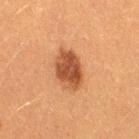workup — total-body-photography surveillance lesion; no biopsy | TBP lesion metrics — an average lesion color of about L≈41 a*≈23 b*≈32 (CIELAB) and about 13 CIELAB-L* units darker than the surrounding skin; border irregularity of about 1.5 on a 0–10 scale, internal color variation of about 4 on a 0–10 scale, and peripheral color asymmetry of about 1.5; a lesion-detection confidence of about 100/100 | subject — female, roughly 40 years of age | lighting — cross-polarized | image — ~15 mm crop, total-body skin-cancer survey | diameter — about 4.5 mm | anatomic site — the right thigh.